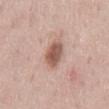notes: imaged on a skin check; not biopsied | lesion size: ~4 mm (longest diameter) | automated lesion analysis: a nevus-likeness score of about 100/100 and lesion-presence confidence of about 100/100 | location: the mid back | image source: ~15 mm crop, total-body skin-cancer survey | patient: male, roughly 40 years of age.